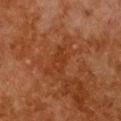notes — imaged on a skin check; not biopsied
acquisition — 15 mm crop, total-body photography
patient — female, aged 48 to 52
site — the chest
automated metrics — a lesion area of about 4 mm² and an outline eccentricity of about 0.85 (0 = round, 1 = elongated); a lesion color around L≈29 a*≈23 b*≈31 in CIELAB and about 4 CIELAB-L* units darker than the surrounding skin; a classifier nevus-likeness of about 0/100 and a lesion-detection confidence of about 100/100
size — ≈3 mm
illumination — cross-polarized illumination